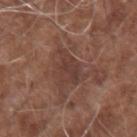workup: total-body-photography surveillance lesion; no biopsy
subject: male, in their mid-70s
anatomic site: the right forearm
image source: ~15 mm tile from a whole-body skin photo
TBP lesion metrics: an area of roughly 8.5 mm²; border irregularity of about 4.5 on a 0–10 scale, a color-variation rating of about 2.5/10, and radial color variation of about 1; a detector confidence of about 90 out of 100 that the crop contains a lesion
size: ~4 mm (longest diameter)
illumination: white-light illumination